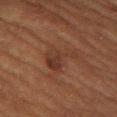No biopsy was performed on this lesion — it was imaged during a full skin examination and was not determined to be concerning.
Automated image analysis of the tile measured an outline eccentricity of about 0.85 (0 = round, 1 = elongated). It also reported a mean CIELAB color near L≈29 a*≈18 b*≈24. The analysis additionally found a classifier nevus-likeness of about 5/100 and a detector confidence of about 100 out of 100 that the crop contains a lesion.
The lesion is located on the chest.
About 5 mm across.
This image is a 15 mm lesion crop taken from a total-body photograph.
A female patient aged approximately 70.
Imaged with cross-polarized lighting.